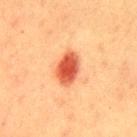Assessment: Part of a total-body skin-imaging series; this lesion was reviewed on a skin check and was not flagged for biopsy. Context: Cropped from a total-body skin-imaging series; the visible field is about 15 mm. About 4 mm across. On the back. The lesion-visualizer software estimated a mean CIELAB color near L≈48 a*≈33 b*≈36, about 16 CIELAB-L* units darker than the surrounding skin, and a lesion-to-skin contrast of about 11 (normalized; higher = more distinct). The software also gave a nevus-likeness score of about 100/100 and lesion-presence confidence of about 100/100. A male subject, approximately 40 years of age.